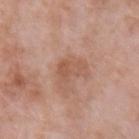  biopsy_status: not biopsied; imaged during a skin examination